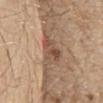The lesion was photographed on a routine skin check and not biopsied; there is no pathology result. Cropped from a whole-body photographic skin survey; the tile spans about 15 mm. A male subject, aged 68 to 72. About 5 mm across. The lesion is located on the chest.